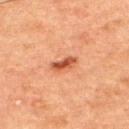Clinical impression: The lesion was photographed on a routine skin check and not biopsied; there is no pathology result. Acquisition and patient details: A male subject roughly 50 years of age. A roughly 15 mm field-of-view crop from a total-body skin photograph. The lesion is located on the upper back.